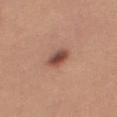Q: Was this lesion biopsied?
A: no biopsy performed (imaged during a skin exam)
Q: What lighting was used for the tile?
A: white-light
Q: Patient demographics?
A: female, in their mid- to late 20s
Q: What kind of image is this?
A: total-body-photography crop, ~15 mm field of view
Q: How large is the lesion?
A: ~3.5 mm (longest diameter)
Q: Where on the body is the lesion?
A: the leg
Q: What did automated image analysis measure?
A: a lesion color around L≈49 a*≈23 b*≈27 in CIELAB, about 13 CIELAB-L* units darker than the surrounding skin, and a lesion-to-skin contrast of about 9.5 (normalized; higher = more distinct); a border-irregularity rating of about 2/10 and internal color variation of about 5.5 on a 0–10 scale